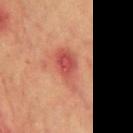- follow-up · total-body-photography surveillance lesion; no biopsy
- patient · male, approximately 70 years of age
- anatomic site · the chest
- image source · total-body-photography crop, ~15 mm field of view